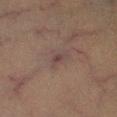Q: Was this lesion biopsied?
A: total-body-photography surveillance lesion; no biopsy
Q: How large is the lesion?
A: ~2.5 mm (longest diameter)
Q: What did automated image analysis measure?
A: a lesion area of about 3 mm² and an outline eccentricity of about 0.8 (0 = round, 1 = elongated); border irregularity of about 4 on a 0–10 scale, a within-lesion color-variation index near 1/10, and radial color variation of about 0.5
Q: What are the patient's age and sex?
A: male, aged 48–52
Q: Lesion location?
A: the right lower leg
Q: How was this image acquired?
A: ~15 mm crop, total-body skin-cancer survey